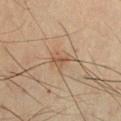<lesion>
<biopsy_status>not biopsied; imaged during a skin examination</biopsy_status>
<lighting>cross-polarized</lighting>
<site>chest</site>
<automated_metrics>
  <cielab_L>44</cielab_L>
  <cielab_a>15</cielab_a>
  <cielab_b>27</cielab_b>
  <vs_skin_darker_L>7.0</vs_skin_darker_L>
  <lesion_detection_confidence_0_100>100</lesion_detection_confidence_0_100>
</automated_metrics>
<image>
  <source>total-body photography crop</source>
  <field_of_view_mm>15</field_of_view_mm>
</image>
<patient>
  <sex>male</sex>
  <age_approx>60</age_approx>
</patient>
</lesion>